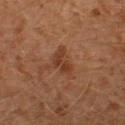Case summary:
* biopsy status · no biopsy performed (imaged during a skin exam)